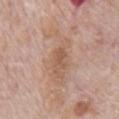workup: total-body-photography surveillance lesion; no biopsy
site: the abdomen
lesion size: ~7 mm (longest diameter)
subject: male, aged 68–72
illumination: white-light
acquisition: 15 mm crop, total-body photography
image-analysis metrics: an area of roughly 16 mm², a shape eccentricity near 0.9, and a symmetry-axis asymmetry near 0.4; a lesion color around L≈59 a*≈18 b*≈29 in CIELAB and a lesion–skin lightness drop of about 7; a classifier nevus-likeness of about 0/100 and a lesion-detection confidence of about 100/100